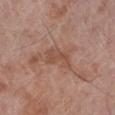The lesion was photographed on a routine skin check and not biopsied; there is no pathology result.
This is a white-light tile.
A region of skin cropped from a whole-body photographic capture, roughly 15 mm wide.
A male patient aged approximately 70.
The lesion is on the left lower leg.
Approximately 4 mm at its widest.